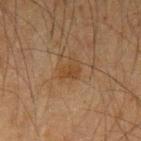Case summary:
- notes · catalogued during a skin exam; not biopsied
- acquisition · ~15 mm crop, total-body skin-cancer survey
- subject · male, in their mid-60s
- size · ≈3 mm
- site · the left upper arm
- TBP lesion metrics · roughly 5 lightness units darker than nearby skin and a normalized border contrast of about 6; a border-irregularity index near 4/10 and a within-lesion color-variation index near 2/10; a classifier nevus-likeness of about 10/100 and lesion-presence confidence of about 100/100
- illumination · cross-polarized illumination